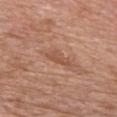Impression:
Recorded during total-body skin imaging; not selected for excision or biopsy.
Image and clinical context:
The lesion-visualizer software estimated a lesion color around L≈52 a*≈23 b*≈31 in CIELAB, about 8 CIELAB-L* units darker than the surrounding skin, and a lesion-to-skin contrast of about 5.5 (normalized; higher = more distinct). The analysis additionally found border irregularity of about 3.5 on a 0–10 scale, internal color variation of about 0.5 on a 0–10 scale, and peripheral color asymmetry of about 0. It also reported a nevus-likeness score of about 0/100 and lesion-presence confidence of about 95/100. The lesion is located on the chest. Cropped from a whole-body photographic skin survey; the tile spans about 15 mm. Imaged with white-light lighting. A female subject, roughly 65 years of age. Longest diameter approximately 3.5 mm.